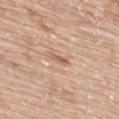follow-up = imaged on a skin check; not biopsied | image source = ~15 mm tile from a whole-body skin photo | site = the upper back | subject = female, in their mid-80s or older | diameter = ~2.5 mm (longest diameter).